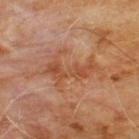| field | value |
|---|---|
| follow-up | imaged on a skin check; not biopsied |
| image-analysis metrics | a lesion area of about 13 mm², an eccentricity of roughly 0.85, and a shape-asymmetry score of about 0.5 (0 = symmetric); border irregularity of about 9 on a 0–10 scale, a color-variation rating of about 3/10, and peripheral color asymmetry of about 1; a nevus-likeness score of about 0/100 and a lesion-detection confidence of about 100/100 |
| diameter | about 6 mm |
| site | the chest |
| patient | male, approximately 60 years of age |
| imaging modality | total-body-photography crop, ~15 mm field of view |
| tile lighting | cross-polarized illumination |